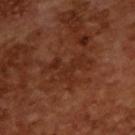Q: Is there a histopathology result?
A: no biopsy performed (imaged during a skin exam)
Q: How was the tile lit?
A: cross-polarized
Q: Patient demographics?
A: male, in their mid-60s
Q: What kind of image is this?
A: 15 mm crop, total-body photography
Q: What did automated image analysis measure?
A: a border-irregularity rating of about 10/10 and a color-variation rating of about 0.5/10; a nevus-likeness score of about 0/100 and a detector confidence of about 100 out of 100 that the crop contains a lesion
Q: Lesion size?
A: about 5 mm